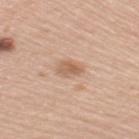Q: Is there a histopathology result?
A: imaged on a skin check; not biopsied
Q: What lighting was used for the tile?
A: white-light
Q: How was this image acquired?
A: ~15 mm crop, total-body skin-cancer survey
Q: Who is the patient?
A: female, roughly 60 years of age
Q: What did automated image analysis measure?
A: a lesion area of about 4.5 mm², an outline eccentricity of about 0.75 (0 = round, 1 = elongated), and two-axis asymmetry of about 0.3; a lesion color around L≈61 a*≈19 b*≈32 in CIELAB, a lesion–skin lightness drop of about 10, and a normalized border contrast of about 6.5; a border-irregularity index near 3/10 and a within-lesion color-variation index near 2.5/10; a classifier nevus-likeness of about 60/100 and lesion-presence confidence of about 100/100
Q: Where on the body is the lesion?
A: the right upper arm